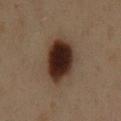Imaged during a routine full-body skin examination; the lesion was not biopsied and no histopathology is available.
A male subject roughly 60 years of age.
On the chest.
A region of skin cropped from a whole-body photographic capture, roughly 15 mm wide.
Automated tile analysis of the lesion measured a mean CIELAB color near L≈25 a*≈15 b*≈21 and a lesion–skin lightness drop of about 17. It also reported a border-irregularity rating of about 1.5/10 and a color-variation rating of about 7/10.
This is a cross-polarized tile.
The recorded lesion diameter is about 6.5 mm.